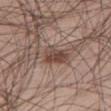Recorded during total-body skin imaging; not selected for excision or biopsy. Located on the right thigh. Longest diameter approximately 3.5 mm. A male subject, aged 53 to 57. A 15 mm close-up extracted from a 3D total-body photography capture. Imaged with white-light lighting.